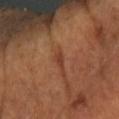Case summary:
– biopsy status — imaged on a skin check; not biopsied
– subject — aged around 60
– site — the right forearm
– automated metrics — a border-irregularity index near 3.5/10, internal color variation of about 0 on a 0–10 scale, and radial color variation of about 0
– image — total-body-photography crop, ~15 mm field of view
– lesion diameter — ≈2.5 mm